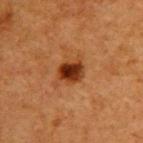Context:
A female subject, aged 38 to 42. Captured under cross-polarized illumination. A lesion tile, about 15 mm wide, cut from a 3D total-body photograph. Longest diameter approximately 3 mm. The lesion-visualizer software estimated a border-irregularity index near 1.5/10, a color-variation rating of about 6/10, and a peripheral color-asymmetry measure near 2. The software also gave a nevus-likeness score of about 100/100 and a lesion-detection confidence of about 100/100. From the upper back.